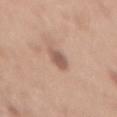tile lighting = white-light illumination
body site = the mid back
subject = female, in their 40s
lesion diameter = about 3 mm
image source = ~15 mm tile from a whole-body skin photo
automated metrics = a mean CIELAB color near L≈56 a*≈19 b*≈26, roughly 11 lightness units darker than nearby skin, and a normalized border contrast of about 7.5; a border-irregularity index near 2.5/10, a color-variation rating of about 1.5/10, and a peripheral color-asymmetry measure near 0.5